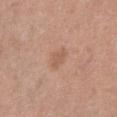Part of a total-body skin-imaging series; this lesion was reviewed on a skin check and was not flagged for biopsy. The lesion-visualizer software estimated roughly 7 lightness units darker than nearby skin and a normalized lesion–skin contrast near 5. The software also gave a border-irregularity rating of about 3/10 and radial color variation of about 0.5. It also reported a classifier nevus-likeness of about 0/100 and lesion-presence confidence of about 100/100. A female subject, in their 40s. The lesion is located on the right thigh. Approximately 3 mm at its widest. A 15 mm close-up extracted from a 3D total-body photography capture.